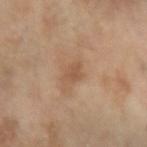<lesion>
<biopsy_status>not biopsied; imaged during a skin examination</biopsy_status>
<site>left forearm</site>
<automated_metrics>
  <border_irregularity_0_10>3.0</border_irregularity_0_10>
  <color_variation_0_10>1.0</color_variation_0_10>
  <peripheral_color_asymmetry>0.5</peripheral_color_asymmetry>
  <nevus_likeness_0_100>0</nevus_likeness_0_100>
  <lesion_detection_confidence_0_100>100</lesion_detection_confidence_0_100>
</automated_metrics>
<lesion_size>
  <long_diameter_mm_approx>2.5</long_diameter_mm_approx>
</lesion_size>
<patient>
  <sex>female</sex>
  <age_approx>70</age_approx>
</patient>
<lighting>cross-polarized</lighting>
<image>
  <source>total-body photography crop</source>
  <field_of_view_mm>15</field_of_view_mm>
</image>
</lesion>